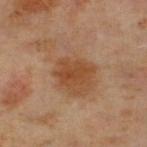This lesion was catalogued during total-body skin photography and was not selected for biopsy. Automated tile analysis of the lesion measured a lesion color around L≈42 a*≈21 b*≈33 in CIELAB, a lesion–skin lightness drop of about 8, and a normalized border contrast of about 8. The analysis additionally found a border-irregularity index near 3/10 and internal color variation of about 2.5 on a 0–10 scale. It also reported a classifier nevus-likeness of about 90/100 and a lesion-detection confidence of about 100/100. Captured under cross-polarized illumination. From the right lower leg. Cropped from a whole-body photographic skin survey; the tile spans about 15 mm. A male subject, approximately 60 years of age. About 4 mm across.